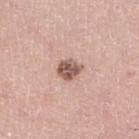This lesion was catalogued during total-body skin photography and was not selected for biopsy.
The lesion is located on the right lower leg.
The recorded lesion diameter is about 3 mm.
Cropped from a total-body skin-imaging series; the visible field is about 15 mm.
A female patient, in their 40s.
This is a white-light tile.